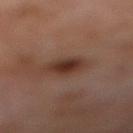Part of a total-body skin-imaging series; this lesion was reviewed on a skin check and was not flagged for biopsy. A 15 mm crop from a total-body photograph taken for skin-cancer surveillance. A male subject about 70 years old. The lesion is located on the right lower leg.